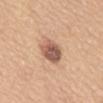Notes:
* workup — no biopsy performed (imaged during a skin exam)
* lesion size — about 4.5 mm
* patient — female, approximately 65 years of age
* image — ~15 mm tile from a whole-body skin photo
* body site — the mid back
* tile lighting — white-light